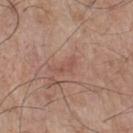workup = catalogued during a skin exam; not biopsied
acquisition = ~15 mm tile from a whole-body skin photo
illumination = white-light illumination
body site = the chest
patient = male, aged approximately 75
lesion size = ~3 mm (longest diameter)